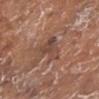biopsy status: imaged on a skin check; not biopsied
subject: female, in their mid-70s
lesion diameter: ≈4.5 mm
site: the left lower leg
acquisition: ~15 mm crop, total-body skin-cancer survey